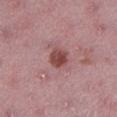biopsy_status: not biopsied; imaged during a skin examination
lesion_size:
  long_diameter_mm_approx: 2.5
image:
  source: total-body photography crop
  field_of_view_mm: 15
patient:
  sex: female
  age_approx: 45
site: leg